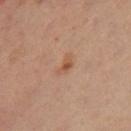Q: Was a biopsy performed?
A: no biopsy performed (imaged during a skin exam)
Q: Lesion size?
A: ~3 mm (longest diameter)
Q: Who is the patient?
A: female, aged approximately 40
Q: Where on the body is the lesion?
A: the left leg
Q: Automated lesion metrics?
A: a mean CIELAB color near L≈55 a*≈21 b*≈33, a lesion–skin lightness drop of about 7, and a normalized border contrast of about 6; a border-irregularity index near 4.5/10, internal color variation of about 3 on a 0–10 scale, and radial color variation of about 1; an automated nevus-likeness rating near 40 out of 100 and a detector confidence of about 100 out of 100 that the crop contains a lesion
Q: How was this image acquired?
A: total-body-photography crop, ~15 mm field of view
Q: How was the tile lit?
A: cross-polarized illumination